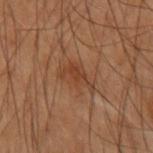  biopsy_status: not biopsied; imaged during a skin examination
  patient:
    sex: male
    age_approx: 55
  image:
    source: total-body photography crop
    field_of_view_mm: 15
  site: right forearm
  automated_metrics:
    cielab_L: 33
    cielab_a: 18
    cielab_b: 27
    vs_skin_contrast_norm: 6.0
    border_irregularity_0_10: 4.0
    peripheral_color_asymmetry: 0.5
    nevus_likeness_0_100: 5
    lesion_detection_confidence_0_100: 100
  lesion_size:
    long_diameter_mm_approx: 4.5
  lighting: cross-polarized